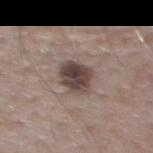Assessment: The lesion was tiled from a total-body skin photograph and was not biopsied. Background: Located on the back. A region of skin cropped from a whole-body photographic capture, roughly 15 mm wide. Approximately 3.5 mm at its widest. Automated image analysis of the tile measured an outline eccentricity of about 0.25 (0 = round, 1 = elongated). A male subject, in their mid- to late 50s. The tile uses white-light illumination.